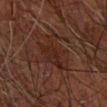Notes:
* follow-up — imaged on a skin check; not biopsied
* location — the right forearm
* imaging modality — ~15 mm crop, total-body skin-cancer survey
* patient — male, aged 68–72